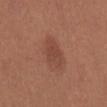Q: Was this lesion biopsied?
A: total-body-photography surveillance lesion; no biopsy
Q: Automated lesion metrics?
A: an average lesion color of about L≈45 a*≈23 b*≈28 (CIELAB) and roughly 7 lightness units darker than nearby skin; border irregularity of about 2.5 on a 0–10 scale and a color-variation rating of about 2.5/10
Q: Who is the patient?
A: female, approximately 25 years of age
Q: How was the tile lit?
A: white-light
Q: What is the anatomic site?
A: the back
Q: How large is the lesion?
A: ~4.5 mm (longest diameter)
Q: What is the imaging modality?
A: ~15 mm crop, total-body skin-cancer survey